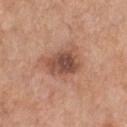{"biopsy_status": "not biopsied; imaged during a skin examination", "lighting": "white-light", "patient": {"sex": "male", "age_approx": 60}, "image": {"source": "total-body photography crop", "field_of_view_mm": 15}, "lesion_size": {"long_diameter_mm_approx": 4.5}, "site": "chest"}